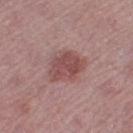follow-up = imaged on a skin check; not biopsied
image source = total-body-photography crop, ~15 mm field of view
site = the leg
lesion size = ≈5 mm
subject = female, in their mid-40s